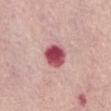Imaged during a routine full-body skin examination; the lesion was not biopsied and no histopathology is available.
On the abdomen.
Cropped from a total-body skin-imaging series; the visible field is about 15 mm.
Automated tile analysis of the lesion measured an eccentricity of roughly 0.3 and a symmetry-axis asymmetry near 0.1. The software also gave a normalized lesion–skin contrast near 12.5. And it measured border irregularity of about 1 on a 0–10 scale, internal color variation of about 5.5 on a 0–10 scale, and peripheral color asymmetry of about 1.5.
Captured under white-light illumination.
A female patient aged approximately 65.